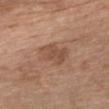Impression: Part of a total-body skin-imaging series; this lesion was reviewed on a skin check and was not flagged for biopsy. Acquisition and patient details: Imaged with white-light lighting. The lesion is located on the chest. Approximately 4 mm at its widest. A 15 mm crop from a total-body photograph taken for skin-cancer surveillance. A male subject aged around 70.